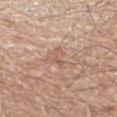Clinical impression: Captured during whole-body skin photography for melanoma surveillance; the lesion was not biopsied. Background: A 15 mm crop from a total-body photograph taken for skin-cancer surveillance. The tile uses white-light illumination. An algorithmic analysis of the crop reported a border-irregularity index near 5/10 and radial color variation of about 1. The lesion is on the left thigh. The subject is a male aged approximately 70. Approximately 3.5 mm at its widest.